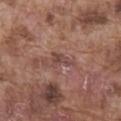No biopsy was performed on this lesion — it was imaged during a full skin examination and was not determined to be concerning. Cropped from a total-body skin-imaging series; the visible field is about 15 mm. Captured under white-light illumination. Measured at roughly 3 mm in maximum diameter. The lesion is on the abdomen. A male subject, about 75 years old.